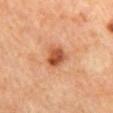| field | value |
|---|---|
| notes | total-body-photography surveillance lesion; no biopsy |
| size | ~3 mm (longest diameter) |
| body site | the back |
| imaging modality | ~15 mm crop, total-body skin-cancer survey |
| automated lesion analysis | a footprint of about 6.5 mm² and a shape-asymmetry score of about 0.2 (0 = symmetric) |
| subject | female, approximately 65 years of age |